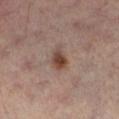Context:
The tile uses cross-polarized illumination. The total-body-photography lesion software estimated an area of roughly 4.5 mm², an outline eccentricity of about 0.75 (0 = round, 1 = elongated), and a symmetry-axis asymmetry near 0.25. It also reported a mean CIELAB color near L≈42 a*≈18 b*≈25 and a lesion–skin lightness drop of about 11. The subject is a male in their 50s. A lesion tile, about 15 mm wide, cut from a 3D total-body photograph. The lesion is located on the left leg. The recorded lesion diameter is about 3 mm.